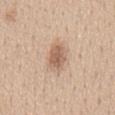| field | value |
|---|---|
| notes | no biopsy performed (imaged during a skin exam) |
| subject | male, about 60 years old |
| anatomic site | the upper back |
| automated lesion analysis | a normalized border contrast of about 7.5; a lesion-detection confidence of about 100/100 |
| image source | ~15 mm tile from a whole-body skin photo |